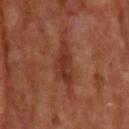This lesion was catalogued during total-body skin photography and was not selected for biopsy.